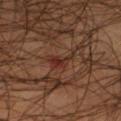Assessment:
Part of a total-body skin-imaging series; this lesion was reviewed on a skin check and was not flagged for biopsy.
Clinical summary:
A 15 mm crop from a total-body photograph taken for skin-cancer surveillance. A male subject about 55 years old. On the right lower leg. The lesion-visualizer software estimated a classifier nevus-likeness of about 0/100 and a detector confidence of about 75 out of 100 that the crop contains a lesion. The recorded lesion diameter is about 3 mm.